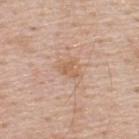• biopsy status · catalogued during a skin exam; not biopsied
• lesion size · ≈3 mm
• patient · male, roughly 50 years of age
• site · the upper back
• image · ~15 mm crop, total-body skin-cancer survey
• automated lesion analysis · an average lesion color of about L≈61 a*≈19 b*≈33 (CIELAB), about 7 CIELAB-L* units darker than the surrounding skin, and a lesion-to-skin contrast of about 6 (normalized; higher = more distinct)